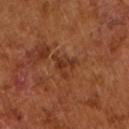This lesion was catalogued during total-body skin photography and was not selected for biopsy. The patient is a male aged 63 to 67. A roughly 15 mm field-of-view crop from a total-body skin photograph. Automated image analysis of the tile measured a lesion area of about 5 mm² and a symmetry-axis asymmetry near 0.55. It also reported an average lesion color of about L≈33 a*≈24 b*≈31 (CIELAB) and a normalized border contrast of about 6.5. It also reported a border-irregularity index near 7/10, internal color variation of about 3 on a 0–10 scale, and radial color variation of about 1. Approximately 3 mm at its widest.